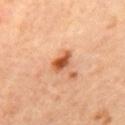Q: Was this lesion biopsied?
A: no biopsy performed (imaged during a skin exam)
Q: Lesion location?
A: the mid back
Q: Lesion size?
A: ~3 mm (longest diameter)
Q: What are the patient's age and sex?
A: female, aged around 45
Q: What kind of image is this?
A: 15 mm crop, total-body photography
Q: What lighting was used for the tile?
A: cross-polarized illumination
Q: What did automated image analysis measure?
A: an average lesion color of about L≈55 a*≈28 b*≈39 (CIELAB), a lesion–skin lightness drop of about 15, and a lesion-to-skin contrast of about 10 (normalized; higher = more distinct)